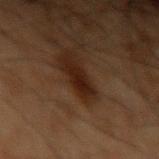Impression:
The lesion was tiled from a total-body skin photograph and was not biopsied.
Clinical summary:
A male patient roughly 50 years of age. This is a cross-polarized tile. From the left upper arm. A 15 mm close-up extracted from a 3D total-body photography capture.